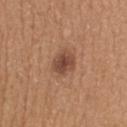Imaged during a routine full-body skin examination; the lesion was not biopsied and no histopathology is available.
Located on the upper back.
Captured under white-light illumination.
The patient is a male about 40 years old.
A roughly 15 mm field-of-view crop from a total-body skin photograph.
The recorded lesion diameter is about 3 mm.